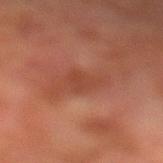follow-up: no biopsy performed (imaged during a skin exam)
subject: male, in their mid-70s
imaging modality: 15 mm crop, total-body photography
location: the left lower leg